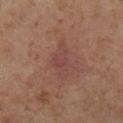Q: Was this lesion biopsied?
A: imaged on a skin check; not biopsied
Q: What lighting was used for the tile?
A: cross-polarized illumination
Q: What is the imaging modality?
A: ~15 mm crop, total-body skin-cancer survey
Q: How large is the lesion?
A: ~5 mm (longest diameter)
Q: What are the patient's age and sex?
A: female, in their mid-50s
Q: What is the anatomic site?
A: the left lower leg